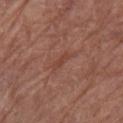follow-up: total-body-photography surveillance lesion; no biopsy.